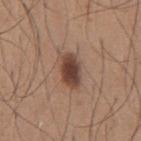Context: About 4 mm across. The lesion is located on the front of the torso. Cropped from a whole-body photographic skin survey; the tile spans about 15 mm. A male subject aged 63 to 67. Captured under white-light illumination.